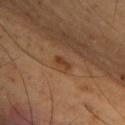follow-up: total-body-photography surveillance lesion; no biopsy
image-analysis metrics: a footprint of about 1.5 mm², an eccentricity of roughly 0.9, and a shape-asymmetry score of about 0.45 (0 = symmetric); a lesion color around L≈36 a*≈21 b*≈31 in CIELAB, roughly 8 lightness units darker than nearby skin, and a normalized lesion–skin contrast near 7.5; a classifier nevus-likeness of about 5/100 and lesion-presence confidence of about 100/100
imaging modality: total-body-photography crop, ~15 mm field of view
subject: male, approximately 50 years of age
location: the upper back
diameter: ≈2 mm
lighting: cross-polarized